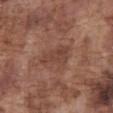Q: Was a biopsy performed?
A: imaged on a skin check; not biopsied
Q: What is the lesion's diameter?
A: ~4 mm (longest diameter)
Q: What are the patient's age and sex?
A: male, aged 73–77
Q: What lighting was used for the tile?
A: white-light
Q: What kind of image is this?
A: ~15 mm tile from a whole-body skin photo
Q: Where on the body is the lesion?
A: the front of the torso